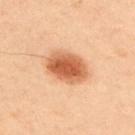Impression: Captured during whole-body skin photography for melanoma surveillance; the lesion was not biopsied. Background: A male patient about 45 years old. This is a cross-polarized tile. A lesion tile, about 15 mm wide, cut from a 3D total-body photograph. Located on the upper back. Approximately 5 mm at its widest.